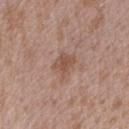Q: How large is the lesion?
A: ≈3 mm
Q: What is the anatomic site?
A: the right upper arm
Q: How was the tile lit?
A: white-light illumination
Q: How was this image acquired?
A: 15 mm crop, total-body photography
Q: Patient demographics?
A: male, about 45 years old